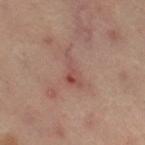| field | value |
|---|---|
| illumination | cross-polarized |
| site | the right thigh |
| lesion diameter | ~4.5 mm (longest diameter) |
| image | ~15 mm crop, total-body skin-cancer survey |
| subject | female, approximately 40 years of age |